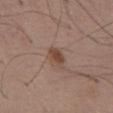The lesion was photographed on a routine skin check and not biopsied; there is no pathology result. Cropped from a whole-body photographic skin survey; the tile spans about 15 mm. From the abdomen. A male patient in their mid-50s. The tile uses white-light illumination. Automated image analysis of the tile measured an area of roughly 5 mm² and a shape eccentricity near 0.65. The software also gave border irregularity of about 2.5 on a 0–10 scale, internal color variation of about 3 on a 0–10 scale, and radial color variation of about 1. The lesion's longest dimension is about 3 mm.